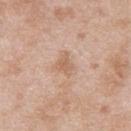Captured during whole-body skin photography for melanoma surveillance; the lesion was not biopsied. About 3 mm across. A male patient in their mid- to late 20s. The lesion is located on the upper back. This is a white-light tile. A close-up tile cropped from a whole-body skin photograph, about 15 mm across.